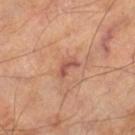notes = catalogued during a skin exam; not biopsied
tile lighting = cross-polarized illumination
imaging modality = ~15 mm crop, total-body skin-cancer survey
site = the left thigh
subject = male, approximately 70 years of age
lesion diameter = ≈2.5 mm